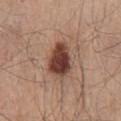<lesion>
<biopsy_status>not biopsied; imaged during a skin examination</biopsy_status>
<lesion_size>
  <long_diameter_mm_approx>4.5</long_diameter_mm_approx>
</lesion_size>
<patient>
  <sex>male</sex>
  <age_approx>45</age_approx>
</patient>
<site>mid back</site>
<lighting>white-light</lighting>
<image>
  <source>total-body photography crop</source>
  <field_of_view_mm>15</field_of_view_mm>
</image>
</lesion>